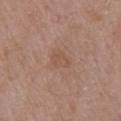Clinical impression:
This lesion was catalogued during total-body skin photography and was not selected for biopsy.
Image and clinical context:
The subject is a male roughly 55 years of age. Approximately 2.5 mm at its widest. A lesion tile, about 15 mm wide, cut from a 3D total-body photograph. The lesion is on the upper back. The tile uses white-light illumination.